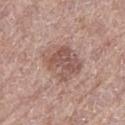notes = catalogued during a skin exam; not biopsied | lesion size = ~4.5 mm (longest diameter) | site = the leg | illumination = white-light | image-analysis metrics = a border-irregularity index near 2/10, internal color variation of about 5 on a 0–10 scale, and peripheral color asymmetry of about 1.5; a nevus-likeness score of about 5/100 and a detector confidence of about 100 out of 100 that the crop contains a lesion | patient = male, aged 68–72 | imaging modality = 15 mm crop, total-body photography.